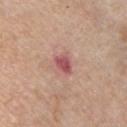  biopsy_status: not biopsied; imaged during a skin examination
  patient:
    sex: male
    age_approx: 60
  image:
    source: total-body photography crop
    field_of_view_mm: 15
  site: chest
  automated_metrics:
    cielab_L: 53
    cielab_a: 29
    cielab_b: 21
    vs_skin_darker_L: 12.0